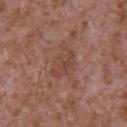Findings:
– follow-up — no biopsy performed (imaged during a skin exam)
– imaging modality — 15 mm crop, total-body photography
– body site — the chest
– subject — male, aged approximately 45